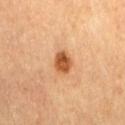Impression:
This lesion was catalogued during total-body skin photography and was not selected for biopsy.
Background:
On the leg. A 15 mm crop from a total-body photograph taken for skin-cancer surveillance. The tile uses cross-polarized illumination. The patient is a female aged 68 to 72. Longest diameter approximately 2.5 mm.